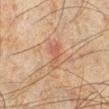Imaged during a routine full-body skin examination; the lesion was not biopsied and no histopathology is available. About 3.5 mm across. A male subject, aged around 45. The tile uses cross-polarized illumination. A roughly 15 mm field-of-view crop from a total-body skin photograph. From the left lower leg.